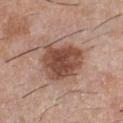| field | value |
|---|---|
| follow-up | total-body-photography surveillance lesion; no biopsy |
| location | the chest |
| size | ≈5.5 mm |
| image | ~15 mm tile from a whole-body skin photo |
| lighting | white-light illumination |
| subject | male, aged approximately 30 |